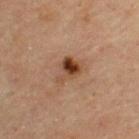Imaged during a routine full-body skin examination; the lesion was not biopsied and no histopathology is available. The tile uses cross-polarized illumination. This image is a 15 mm lesion crop taken from a total-body photograph. The patient is a male aged approximately 45. Approximately 3.5 mm at its widest. On the upper back.